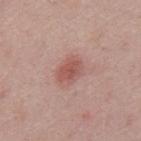biopsy_status: not biopsied; imaged during a skin examination
patient:
  sex: male
  age_approx: 45
image:
  source: total-body photography crop
  field_of_view_mm: 15
lighting: white-light
automated_metrics:
  area_mm2_approx: 6.0
  eccentricity: 0.75
  shape_asymmetry: 0.25
  cielab_L: 54
  cielab_a: 25
  cielab_b: 24
  vs_skin_darker_L: 10.0
  vs_skin_contrast_norm: 7.0
  border_irregularity_0_10: 2.5
  color_variation_0_10: 3.0
  peripheral_color_asymmetry: 1.0
  nevus_likeness_0_100: 70
site: back
lesion_size:
  long_diameter_mm_approx: 3.5